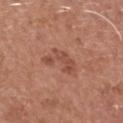Case summary:
• workup — catalogued during a skin exam; not biopsied
• anatomic site — the head or neck
• image source — total-body-photography crop, ~15 mm field of view
• patient — male, aged around 55
• tile lighting — white-light
• size — ~4 mm (longest diameter)